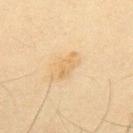notes = total-body-photography surveillance lesion; no biopsy | diameter = about 3.5 mm | subject = male, roughly 35 years of age | image = total-body-photography crop, ~15 mm field of view | site = the back.